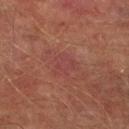location: the right lower leg | lighting: cross-polarized | imaging modality: 15 mm crop, total-body photography | diameter: about 3 mm | patient: male, approximately 75 years of age.